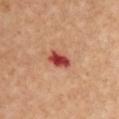biopsy_status: not biopsied; imaged during a skin examination
automated_metrics:
  border_irregularity_0_10: 2.5
  color_variation_0_10: 4.0
  peripheral_color_asymmetry: 1.0
  nevus_likeness_0_100: 0
  lesion_detection_confidence_0_100: 100
site: left upper arm
image:
  source: total-body photography crop
  field_of_view_mm: 15
lighting: cross-polarized
lesion_size:
  long_diameter_mm_approx: 3.0
patient:
  sex: female
  age_approx: 45